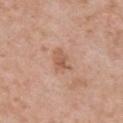Clinical impression: Part of a total-body skin-imaging series; this lesion was reviewed on a skin check and was not flagged for biopsy. Clinical summary: The tile uses white-light illumination. From the chest. A lesion tile, about 15 mm wide, cut from a 3D total-body photograph. The lesion-visualizer software estimated a shape eccentricity near 0.75 and a shape-asymmetry score of about 0.3 (0 = symmetric). And it measured an average lesion color of about L≈57 a*≈22 b*≈31 (CIELAB), about 9 CIELAB-L* units darker than the surrounding skin, and a normalized border contrast of about 6. And it measured border irregularity of about 3 on a 0–10 scale and radial color variation of about 1. The analysis additionally found a nevus-likeness score of about 5/100 and a lesion-detection confidence of about 100/100. The subject is a female aged 38 to 42. Approximately 3 mm at its widest.